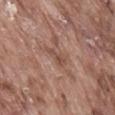biopsy_status: not biopsied; imaged during a skin examination